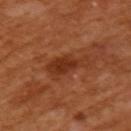Q: Where on the body is the lesion?
A: the left upper arm
Q: How was the tile lit?
A: cross-polarized illumination
Q: What are the patient's age and sex?
A: female, approximately 55 years of age
Q: Lesion size?
A: about 4.5 mm
Q: What is the imaging modality?
A: ~15 mm crop, total-body skin-cancer survey